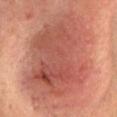biopsy status: no biopsy performed (imaged during a skin exam)
image source: ~15 mm crop, total-body skin-cancer survey
body site: the head or neck
subject: female, aged 63–67